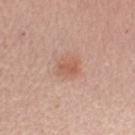Captured during whole-body skin photography for melanoma surveillance; the lesion was not biopsied. On the left forearm. About 2.5 mm across. A female subject approximately 50 years of age. A roughly 15 mm field-of-view crop from a total-body skin photograph.